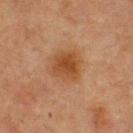notes: catalogued during a skin exam; not biopsied
subject: male, aged 58 to 62
anatomic site: the upper back
diameter: ≈4.5 mm
acquisition: ~15 mm crop, total-body skin-cancer survey
TBP lesion metrics: an eccentricity of roughly 0.55 and a symmetry-axis asymmetry near 0.25; a border-irregularity rating of about 2.5/10 and peripheral color asymmetry of about 1; a lesion-detection confidence of about 100/100
tile lighting: cross-polarized illumination